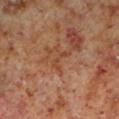– imaging modality · ~15 mm crop, total-body skin-cancer survey
– automated lesion analysis · an outline eccentricity of about 0.9 (0 = round, 1 = elongated); a lesion color around L≈42 a*≈22 b*≈31 in CIELAB, a lesion–skin lightness drop of about 6, and a lesion-to-skin contrast of about 5 (normalized; higher = more distinct); a border-irregularity index near 6/10 and radial color variation of about 0; an automated nevus-likeness rating near 0 out of 100 and a detector confidence of about 95 out of 100 that the crop contains a lesion
– illumination · cross-polarized
– diameter · ~3 mm (longest diameter)
– patient · male, aged 58–62
– location · the leg